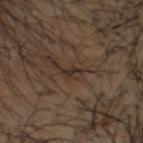biopsy status: catalogued during a skin exam; not biopsied
patient: male, aged 43 to 47
image source: 15 mm crop, total-body photography
image-analysis metrics: a footprint of about 1.5 mm², an outline eccentricity of about 0.95 (0 = round, 1 = elongated), and a symmetry-axis asymmetry near 0.55
location: the head or neck
diameter: about 2.5 mm
lighting: cross-polarized illumination